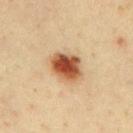This lesion was catalogued during total-body skin photography and was not selected for biopsy.
A male subject, roughly 40 years of age.
Automated image analysis of the tile measured a lesion area of about 11 mm², a shape eccentricity near 0.6, and a shape-asymmetry score of about 0.1 (0 = symmetric). The analysis additionally found an average lesion color of about L≈48 a*≈22 b*≈33 (CIELAB), roughly 17 lightness units darker than nearby skin, and a normalized lesion–skin contrast near 12. It also reported lesion-presence confidence of about 100/100.
The lesion is on the back.
A 15 mm close-up extracted from a 3D total-body photography capture.
Captured under cross-polarized illumination.